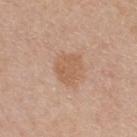Impression:
Recorded during total-body skin imaging; not selected for excision or biopsy.
Image and clinical context:
A female subject, aged 38 to 42. A lesion tile, about 15 mm wide, cut from a 3D total-body photograph. Approximately 3.5 mm at its widest. The lesion is located on the back. Automated tile analysis of the lesion measured an outline eccentricity of about 0.6 (0 = round, 1 = elongated) and a symmetry-axis asymmetry near 0.25. The software also gave a border-irregularity index near 2.5/10, a color-variation rating of about 1.5/10, and a peripheral color-asymmetry measure near 0.5. Imaged with white-light lighting.